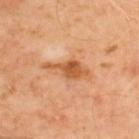Imaged with cross-polarized lighting.
A 15 mm crop from a total-body photograph taken for skin-cancer surveillance.
The patient is a male aged 48 to 52.
On the upper back.